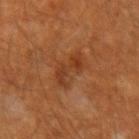<case>
<biopsy_status>not biopsied; imaged during a skin examination</biopsy_status>
<patient>
  <sex>male</sex>
  <age_approx>60</age_approx>
</patient>
<lighting>cross-polarized</lighting>
<image>
  <source>total-body photography crop</source>
  <field_of_view_mm>15</field_of_view_mm>
</image>
<site>right forearm</site>
<lesion_size>
  <long_diameter_mm_approx>4.5</long_diameter_mm_approx>
</lesion_size>
</case>